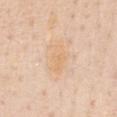follow-up — catalogued during a skin exam; not biopsied
site — the chest
size — about 4 mm
patient — female, aged around 60
imaging modality — 15 mm crop, total-body photography
image-analysis metrics — a lesion area of about 8 mm², an outline eccentricity of about 0.8 (0 = round, 1 = elongated), and two-axis asymmetry of about 0.35; a border-irregularity rating of about 4/10 and a within-lesion color-variation index near 2/10; a lesion-detection confidence of about 100/100